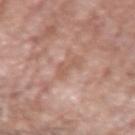Imaged with white-light lighting.
A 15 mm close-up tile from a total-body photography series done for melanoma screening.
A male subject, roughly 80 years of age.
Located on the left upper arm.